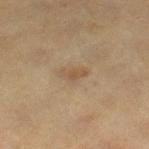Recorded during total-body skin imaging; not selected for excision or biopsy. The recorded lesion diameter is about 3 mm. A region of skin cropped from a whole-body photographic capture, roughly 15 mm wide. The lesion is on the right thigh. A female patient approximately 40 years of age. This is a cross-polarized tile.